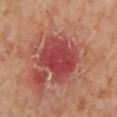Notes:
• notes · catalogued during a skin exam; not biopsied
• size · ~5.5 mm (longest diameter)
• anatomic site · the left lower leg
• subject · female, aged 53 to 57
• image · total-body-photography crop, ~15 mm field of view